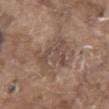| field | value |
|---|---|
| lesion diameter | ≈4.5 mm |
| subject | male, about 80 years old |
| image | ~15 mm crop, total-body skin-cancer survey |
| location | the chest |
| lighting | white-light illumination |